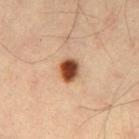Part of a total-body skin-imaging series; this lesion was reviewed on a skin check and was not flagged for biopsy.
Cropped from a whole-body photographic skin survey; the tile spans about 15 mm.
Captured under cross-polarized illumination.
Approximately 3 mm at its widest.
A male patient aged 38–42.
The lesion is located on the left thigh.